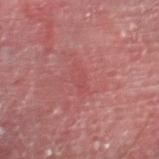{"biopsy_status": "not biopsied; imaged during a skin examination", "patient": {"sex": "male", "age_approx": 55}, "lesion_size": {"long_diameter_mm_approx": 3.0}, "image": {"source": "total-body photography crop", "field_of_view_mm": 15}, "site": "right thigh"}